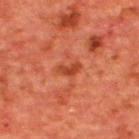Q: Was a biopsy performed?
A: imaged on a skin check; not biopsied